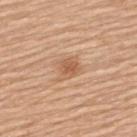Recorded during total-body skin imaging; not selected for excision or biopsy.
Imaged with white-light lighting.
A female subject, approximately 60 years of age.
A lesion tile, about 15 mm wide, cut from a 3D total-body photograph.
On the upper back.
The lesion's longest dimension is about 3 mm.